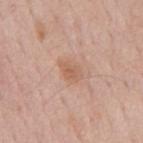Q: Is there a histopathology result?
A: total-body-photography surveillance lesion; no biopsy
Q: Where on the body is the lesion?
A: the mid back
Q: What kind of image is this?
A: 15 mm crop, total-body photography
Q: What are the patient's age and sex?
A: male, approximately 60 years of age
Q: Lesion size?
A: ≈3 mm
Q: Illumination type?
A: white-light illumination